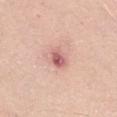Assessment:
Recorded during total-body skin imaging; not selected for excision or biopsy.
Background:
The subject is a female aged 28–32. From the abdomen. A close-up tile cropped from a whole-body skin photograph, about 15 mm across. This is a white-light tile.